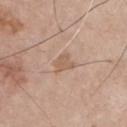Q: Was this lesion biopsied?
A: imaged on a skin check; not biopsied
Q: What is the lesion's diameter?
A: about 2.5 mm
Q: What kind of image is this?
A: 15 mm crop, total-body photography
Q: Patient demographics?
A: male, in their mid-70s
Q: What lighting was used for the tile?
A: white-light illumination
Q: Lesion location?
A: the chest